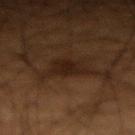follow-up = no biopsy performed (imaged during a skin exam)
tile lighting = cross-polarized illumination
patient = male, roughly 50 years of age
image-analysis metrics = an area of roughly 8 mm², a shape eccentricity near 0.55, and a symmetry-axis asymmetry near 0.3; about 5 CIELAB-L* units darker than the surrounding skin and a normalized border contrast of about 8; a border-irregularity rating of about 3/10, internal color variation of about 2.5 on a 0–10 scale, and peripheral color asymmetry of about 1; a classifier nevus-likeness of about 0/100
acquisition = ~15 mm crop, total-body skin-cancer survey
lesion size = ≈3.5 mm
location = the right forearm